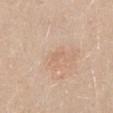notes: catalogued during a skin exam; not biopsied | automated metrics: a lesion color around L≈64 a*≈18 b*≈32 in CIELAB and a normalized lesion–skin contrast near 3.5; lesion-presence confidence of about 100/100 | imaging modality: ~15 mm crop, total-body skin-cancer survey | body site: the mid back | subject: female, aged 38–42 | diameter: about 1 mm.